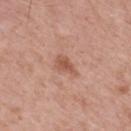Part of a total-body skin-imaging series; this lesion was reviewed on a skin check and was not flagged for biopsy. The tile uses white-light illumination. The lesion is located on the back. Cropped from a total-body skin-imaging series; the visible field is about 15 mm. A male subject about 65 years old. Approximately 3 mm at its widest.